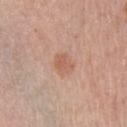notes=no biopsy performed (imaged during a skin exam) | location=the left upper arm | size=≈2.5 mm | subject=female, aged approximately 65 | automated lesion analysis=a footprint of about 4.5 mm², a shape eccentricity near 0.55, and two-axis asymmetry of about 0.25; an average lesion color of about L≈59 a*≈23 b*≈30 (CIELAB), about 7 CIELAB-L* units darker than the surrounding skin, and a normalized lesion–skin contrast near 5.5; lesion-presence confidence of about 100/100 | image=total-body-photography crop, ~15 mm field of view | tile lighting=white-light illumination.